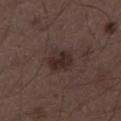biopsy status: imaged on a skin check; not biopsied.